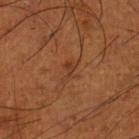{
  "biopsy_status": "not biopsied; imaged during a skin examination",
  "lesion_size": {
    "long_diameter_mm_approx": 2.5
  },
  "patient": {
    "sex": "male",
    "age_approx": 65
  },
  "site": "left lower leg",
  "image": {
    "source": "total-body photography crop",
    "field_of_view_mm": 15
  },
  "lighting": "cross-polarized"
}